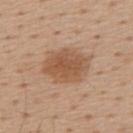Part of a total-body skin-imaging series; this lesion was reviewed on a skin check and was not flagged for biopsy.
A male subject, about 55 years old.
The lesion's longest dimension is about 5.5 mm.
From the mid back.
A roughly 15 mm field-of-view crop from a total-body skin photograph.